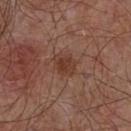Assessment:
Part of a total-body skin-imaging series; this lesion was reviewed on a skin check and was not flagged for biopsy.
Image and clinical context:
The tile uses white-light illumination. A 15 mm close-up tile from a total-body photography series done for melanoma screening. A male patient in their mid- to late 50s. On the chest.